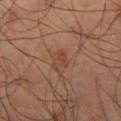biopsy_status: not biopsied; imaged during a skin examination
image:
  source: total-body photography crop
  field_of_view_mm: 15
lesion_size:
  long_diameter_mm_approx: 3.0
patient:
  sex: male
  age_approx: 65
site: right thigh
lighting: cross-polarized